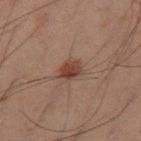No biopsy was performed on this lesion — it was imaged during a full skin examination and was not determined to be concerning.
A male patient aged 58–62.
A region of skin cropped from a whole-body photographic capture, roughly 15 mm wide.
From the leg.
This is a cross-polarized tile.